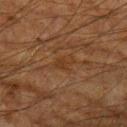Impression:
The lesion was tiled from a total-body skin photograph and was not biopsied.
Acquisition and patient details:
From the left upper arm. Imaged with cross-polarized lighting. A male patient aged approximately 65. A 15 mm close-up tile from a total-body photography series done for melanoma screening.